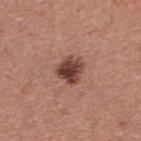biopsy status=imaged on a skin check; not biopsied | lesion size=≈3 mm | patient=male, aged 38–42 | location=the upper back | image source=~15 mm crop, total-body skin-cancer survey.